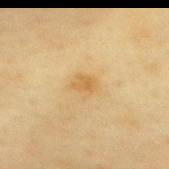Notes:
– follow-up — catalogued during a skin exam; not biopsied
– anatomic site — the mid back
– illumination — cross-polarized illumination
– imaging modality — ~15 mm tile from a whole-body skin photo
– lesion size — ~2.5 mm (longest diameter)
– TBP lesion metrics — an area of roughly 3.5 mm², a shape eccentricity near 0.75, and a shape-asymmetry score of about 0.35 (0 = symmetric); a lesion color around L≈59 a*≈15 b*≈42 in CIELAB and a normalized border contrast of about 6; a nevus-likeness score of about 20/100 and a lesion-detection confidence of about 100/100
– patient — female, roughly 60 years of age